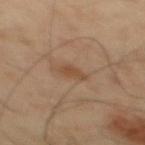notes: no biopsy performed (imaged during a skin exam) | diameter: ~2.5 mm (longest diameter) | tile lighting: cross-polarized | site: the mid back | subject: male, in their 40s | image source: total-body-photography crop, ~15 mm field of view | TBP lesion metrics: a border-irregularity index near 3/10, a within-lesion color-variation index near 1.5/10, and radial color variation of about 0.5; a classifier nevus-likeness of about 50/100 and lesion-presence confidence of about 100/100.